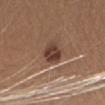Part of a total-body skin-imaging series; this lesion was reviewed on a skin check and was not flagged for biopsy. Captured under white-light illumination. A region of skin cropped from a whole-body photographic capture, roughly 15 mm wide. The lesion is on the head or neck. A female patient, roughly 20 years of age. The lesion-visualizer software estimated about 11 CIELAB-L* units darker than the surrounding skin and a lesion-to-skin contrast of about 9.5 (normalized; higher = more distinct). The analysis additionally found a within-lesion color-variation index near 5/10 and radial color variation of about 1.5. Longest diameter approximately 3.5 mm.